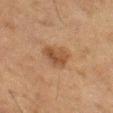biopsy_status: not biopsied; imaged during a skin examination
lesion_size:
  long_diameter_mm_approx: 3.5
lighting: cross-polarized
site: left thigh
automated_metrics:
  vs_skin_darker_L: 8.0
  vs_skin_contrast_norm: 7.0
  border_irregularity_0_10: 2.5
  color_variation_0_10: 3.0
  peripheral_color_asymmetry: 1.0
  nevus_likeness_0_100: 75
image:
  source: total-body photography crop
  field_of_view_mm: 15
patient:
  sex: male
  age_approx: 75